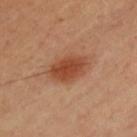Q: Was this lesion biopsied?
A: total-body-photography surveillance lesion; no biopsy
Q: Lesion size?
A: ≈5.5 mm
Q: What kind of image is this?
A: total-body-photography crop, ~15 mm field of view
Q: Who is the patient?
A: male, aged around 40
Q: Lesion location?
A: the chest
Q: Automated lesion metrics?
A: an eccentricity of roughly 0.8 and two-axis asymmetry of about 0.15; a mean CIELAB color near L≈47 a*≈25 b*≈34 and a lesion–skin lightness drop of about 10; a border-irregularity index near 2/10 and radial color variation of about 1; a nevus-likeness score of about 100/100 and a lesion-detection confidence of about 100/100